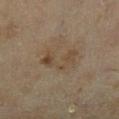Impression:
Imaged during a routine full-body skin examination; the lesion was not biopsied and no histopathology is available.
Context:
On the left lower leg. A female subject about 60 years old. A close-up tile cropped from a whole-body skin photograph, about 15 mm across.